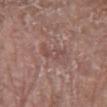The lesion was tiled from a total-body skin photograph and was not biopsied. The lesion is located on the left forearm. Approximately 3 mm at its widest. A female patient in their 70s. Automated image analysis of the tile measured a symmetry-axis asymmetry near 0.45. The software also gave border irregularity of about 5 on a 0–10 scale and radial color variation of about 0. It also reported a classifier nevus-likeness of about 0/100. A 15 mm close-up tile from a total-body photography series done for melanoma screening. Captured under white-light illumination.